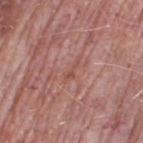biopsy status — no biopsy performed (imaged during a skin exam) | automated lesion analysis — an outline eccentricity of about 0.95 (0 = round, 1 = elongated) and two-axis asymmetry of about 0.65; internal color variation of about 0 on a 0–10 scale | imaging modality — total-body-photography crop, ~15 mm field of view | lesion diameter — ≈2.5 mm | tile lighting — white-light illumination | subject — female, aged around 70 | location — the leg.